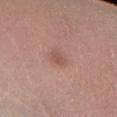biopsy status: catalogued during a skin exam; not biopsied | patient: male, roughly 55 years of age | anatomic site: the left lower leg | imaging modality: 15 mm crop, total-body photography | lighting: cross-polarized | automated metrics: a classifier nevus-likeness of about 20/100 and a detector confidence of about 100 out of 100 that the crop contains a lesion.